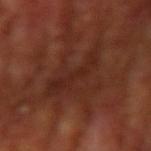The lesion was photographed on a routine skin check and not biopsied; there is no pathology result. From the right upper arm. The subject is a male approximately 65 years of age. Cropped from a total-body skin-imaging series; the visible field is about 15 mm.